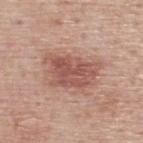workup = no biopsy performed (imaged during a skin exam) | lesion diameter = ≈6 mm | anatomic site = the upper back | subject = male, roughly 75 years of age | image source = total-body-photography crop, ~15 mm field of view.